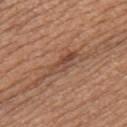This lesion was catalogued during total-body skin photography and was not selected for biopsy. A 15 mm close-up extracted from a 3D total-body photography capture. The patient is a female aged approximately 45. Captured under white-light illumination. Located on the upper back.